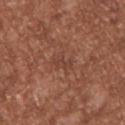This lesion was catalogued during total-body skin photography and was not selected for biopsy. The recorded lesion diameter is about 3 mm. From the back. A male subject aged around 45. Captured under white-light illumination. A 15 mm crop from a total-body photograph taken for skin-cancer surveillance.